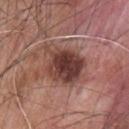workup: catalogued during a skin exam; not biopsied | TBP lesion metrics: an area of roughly 18 mm² | acquisition: ~15 mm crop, total-body skin-cancer survey | patient: male, aged 43–47 | illumination: white-light | body site: the upper back | lesion diameter: ~5.5 mm (longest diameter).